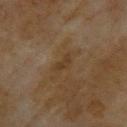| key | value |
|---|---|
| notes | catalogued during a skin exam; not biopsied |
| site | the head or neck |
| automated metrics | a lesion color around L≈33 a*≈14 b*≈29 in CIELAB, a lesion–skin lightness drop of about 6, and a lesion-to-skin contrast of about 6 (normalized; higher = more distinct); a nevus-likeness score of about 0/100 and a lesion-detection confidence of about 95/100 |
| diameter | ~2.5 mm (longest diameter) |
| image | ~15 mm crop, total-body skin-cancer survey |
| subject | female, in their 60s |